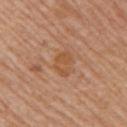Q: Is there a histopathology result?
A: no biopsy performed (imaged during a skin exam)
Q: What is the imaging modality?
A: 15 mm crop, total-body photography
Q: Lesion location?
A: the right upper arm
Q: What is the lesion's diameter?
A: ~3 mm (longest diameter)
Q: How was the tile lit?
A: white-light illumination
Q: What did automated image analysis measure?
A: an automated nevus-likeness rating near 0 out of 100 and lesion-presence confidence of about 100/100
Q: Patient demographics?
A: female, aged approximately 55